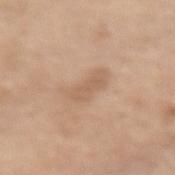No biopsy was performed on this lesion — it was imaged during a full skin examination and was not determined to be concerning. The lesion is on the lower back. Measured at roughly 4 mm in maximum diameter. Captured under white-light illumination. This image is a 15 mm lesion crop taken from a total-body photograph. The patient is a male aged around 70. An algorithmic analysis of the crop reported a lesion color around L≈61 a*≈17 b*≈32 in CIELAB, a lesion–skin lightness drop of about 7, and a normalized lesion–skin contrast near 5. It also reported border irregularity of about 4 on a 0–10 scale, a within-lesion color-variation index near 1.5/10, and radial color variation of about 0.5.